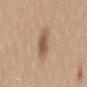follow-up = no biopsy performed (imaged during a skin exam)
body site = the lower back
subject = female, aged 28–32
diameter = about 3.5 mm
image = ~15 mm tile from a whole-body skin photo